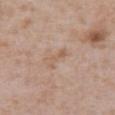The lesion was tiled from a total-body skin photograph and was not biopsied.